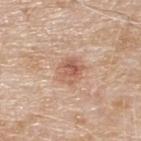The lesion was tiled from a total-body skin photograph and was not biopsied. A roughly 15 mm field-of-view crop from a total-body skin photograph. Imaged with white-light lighting. Approximately 3 mm at its widest. A male patient approximately 80 years of age. From the upper back.